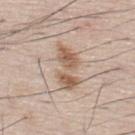Notes:
– workup · no biopsy performed (imaged during a skin exam)
– lighting · white-light
– image source · ~15 mm crop, total-body skin-cancer survey
– subject · male, in their mid-70s
– size · ~5.5 mm (longest diameter)
– location · the upper back